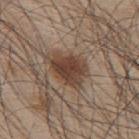Recorded during total-body skin imaging; not selected for excision or biopsy. A 15 mm close-up tile from a total-body photography series done for melanoma screening. The patient is a male aged approximately 45. Approximately 4.5 mm at its widest. The lesion-visualizer software estimated a lesion area of about 11 mm², an outline eccentricity of about 0.7 (0 = round, 1 = elongated), and a shape-asymmetry score of about 0.3 (0 = symmetric). The lesion is located on the mid back.